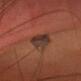notes=catalogued during a skin exam; not biopsied